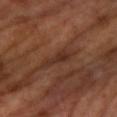- biopsy status · imaged on a skin check; not biopsied
- patient · female, in their 70s
- size · ≈4 mm
- body site · the right forearm
- lighting · cross-polarized
- image source · ~15 mm crop, total-body skin-cancer survey